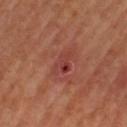The patient is a female aged 63–67.
Captured under cross-polarized illumination.
A roughly 15 mm field-of-view crop from a total-body skin photograph.
Automated tile analysis of the lesion measured a lesion–skin lightness drop of about 7 and a normalized border contrast of about 6. And it measured a border-irregularity index near 4/10, a within-lesion color-variation index near 6.5/10, and a peripheral color-asymmetry measure near 2. It also reported a nevus-likeness score of about 0/100 and lesion-presence confidence of about 100/100.
The lesion is located on the left thigh.
Longest diameter approximately 4 mm.